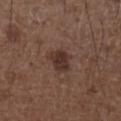biopsy status = imaged on a skin check; not biopsied | site = the right upper arm | subject = male, roughly 50 years of age | tile lighting = white-light | acquisition = ~15 mm crop, total-body skin-cancer survey | lesion diameter = ~3 mm (longest diameter).